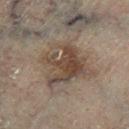This lesion was catalogued during total-body skin photography and was not selected for biopsy.
Approximately 6 mm at its widest.
The lesion is on the left lower leg.
The subject is a male aged around 70.
A region of skin cropped from a whole-body photographic capture, roughly 15 mm wide.